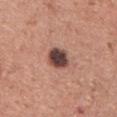Notes:
* notes: catalogued during a skin exam; not biopsied
* image: total-body-photography crop, ~15 mm field of view
* anatomic site: the chest
* patient: male, in their mid- to late 40s
* tile lighting: white-light
* size: about 3 mm
* automated lesion analysis: a footprint of about 6.5 mm², an outline eccentricity of about 0.45 (0 = round, 1 = elongated), and a shape-asymmetry score of about 0.15 (0 = symmetric); a border-irregularity rating of about 1.5/10, a color-variation rating of about 5/10, and radial color variation of about 1; a nevus-likeness score of about 20/100 and a lesion-detection confidence of about 100/100